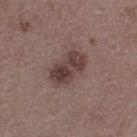biopsy_status: not biopsied; imaged during a skin examination
site: left thigh
image:
  source: total-body photography crop
  field_of_view_mm: 15
patient:
  sex: female
  age_approx: 50
automated_metrics:
  cielab_L: 39
  cielab_a: 17
  cielab_b: 19
  vs_skin_darker_L: 11.0
  vs_skin_contrast_norm: 9.0
  border_irregularity_0_10: 2.5
  color_variation_0_10: 5.0
  nevus_likeness_0_100: 65
  lesion_detection_confidence_0_100: 100